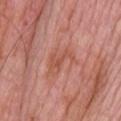Impression: Captured during whole-body skin photography for melanoma surveillance; the lesion was not biopsied. Background: About 4.5 mm across. The subject is a male in their mid- to late 60s. This image is a 15 mm lesion crop taken from a total-body photograph. On the upper back. The total-body-photography lesion software estimated a border-irregularity index near 6/10, a within-lesion color-variation index near 3.5/10, and peripheral color asymmetry of about 1. And it measured a lesion-detection confidence of about 100/100. The tile uses white-light illumination.